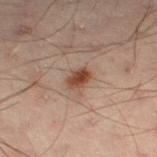Q: Was this lesion biopsied?
A: total-body-photography surveillance lesion; no biopsy
Q: What is the anatomic site?
A: the left lower leg
Q: Patient demographics?
A: male, approximately 50 years of age
Q: How was this image acquired?
A: ~15 mm crop, total-body skin-cancer survey
Q: What is the lesion's diameter?
A: ≈2.5 mm